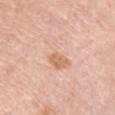follow-up: imaged on a skin check; not biopsied
imaging modality: total-body-photography crop, ~15 mm field of view
location: the left upper arm
patient: female, roughly 65 years of age